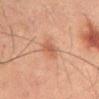The lesion was tiled from a total-body skin photograph and was not biopsied.
Automated image analysis of the tile measured a shape-asymmetry score of about 0.25 (0 = symmetric). The analysis additionally found an average lesion color of about L≈50 a*≈22 b*≈29 (CIELAB) and a normalized border contrast of about 6. The analysis additionally found border irregularity of about 3 on a 0–10 scale and internal color variation of about 1.5 on a 0–10 scale. It also reported a nevus-likeness score of about 35/100 and a lesion-detection confidence of about 100/100.
The tile uses cross-polarized illumination.
Cropped from a total-body skin-imaging series; the visible field is about 15 mm.
The recorded lesion diameter is about 3.5 mm.
On the abdomen.
A male patient, roughly 55 years of age.